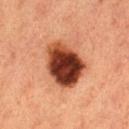Part of a total-body skin-imaging series; this lesion was reviewed on a skin check and was not flagged for biopsy.
From the right thigh.
Captured under cross-polarized illumination.
A female patient in their 40s.
A lesion tile, about 15 mm wide, cut from a 3D total-body photograph.